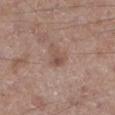Approximately 2.5 mm at its widest. The tile uses white-light illumination. The patient is a male in their mid- to late 60s. An algorithmic analysis of the crop reported a footprint of about 4 mm², an eccentricity of roughly 0.65, and a symmetry-axis asymmetry near 0.5. A roughly 15 mm field-of-view crop from a total-body skin photograph. Located on the leg.